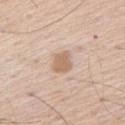| field | value |
|---|---|
| workup | no biopsy performed (imaged during a skin exam) |
| site | the left upper arm |
| subject | male, roughly 70 years of age |
| imaging modality | ~15 mm tile from a whole-body skin photo |